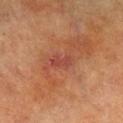Assessment:
Part of a total-body skin-imaging series; this lesion was reviewed on a skin check and was not flagged for biopsy.